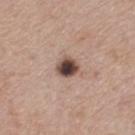• workup: catalogued during a skin exam; not biopsied
• image source: ~15 mm tile from a whole-body skin photo
• subject: male, aged 53 to 57
• automated lesion analysis: a lesion color around L≈45 a*≈16 b*≈22 in CIELAB, roughly 20 lightness units darker than nearby skin, and a lesion-to-skin contrast of about 13.5 (normalized; higher = more distinct); a border-irregularity index near 1.5/10 and a peripheral color-asymmetry measure near 1.5
• illumination: white-light illumination